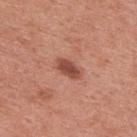• notes: no biopsy performed (imaged during a skin exam)
• automated metrics: a lesion area of about 5 mm² and an outline eccentricity of about 0.85 (0 = round, 1 = elongated); a border-irregularity index near 2.5/10 and a color-variation rating of about 2.5/10
• subject: male, aged approximately 55
• acquisition: 15 mm crop, total-body photography
• location: the upper back
• lesion size: about 3.5 mm
• tile lighting: white-light